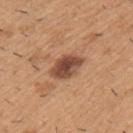Assessment:
Imaged during a routine full-body skin examination; the lesion was not biopsied and no histopathology is available.
Context:
A male subject, aged approximately 40. Located on the left upper arm. This image is a 15 mm lesion crop taken from a total-body photograph.